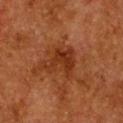Findings:
• workup — imaged on a skin check; not biopsied
• automated lesion analysis — border irregularity of about 6 on a 0–10 scale, a within-lesion color-variation index near 2.5/10, and radial color variation of about 0.5; an automated nevus-likeness rating near 0 out of 100 and a lesion-detection confidence of about 100/100
• patient — female, approximately 50 years of age
• size — ≈4 mm
• image — ~15 mm tile from a whole-body skin photo
• body site — the chest